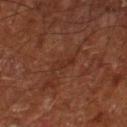Notes:
– workup — imaged on a skin check; not biopsied
– image source — ~15 mm tile from a whole-body skin photo
– image-analysis metrics — a footprint of about 3.5 mm², an eccentricity of roughly 0.9, and two-axis asymmetry of about 0.35; a nevus-likeness score of about 0/100 and lesion-presence confidence of about 60/100
– lesion diameter — about 3 mm
– subject — male, aged 63 to 67
– anatomic site — the right lower leg